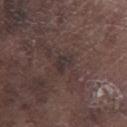This image is a 15 mm lesion crop taken from a total-body photograph.
This is a white-light tile.
An algorithmic analysis of the crop reported a lesion area of about 3.5 mm², an outline eccentricity of about 0.7 (0 = round, 1 = elongated), and a symmetry-axis asymmetry near 0.4. It also reported border irregularity of about 3.5 on a 0–10 scale, internal color variation of about 3 on a 0–10 scale, and peripheral color asymmetry of about 1. And it measured lesion-presence confidence of about 65/100.
The subject is a male about 75 years old.
From the right lower leg.
The recorded lesion diameter is about 2.5 mm.